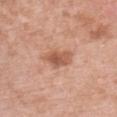Findings:
• biopsy status · no biopsy performed (imaged during a skin exam)
• patient · female, about 50 years old
• acquisition · ~15 mm crop, total-body skin-cancer survey
• anatomic site · the chest
• diameter · about 4 mm